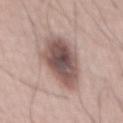Assessment: Recorded during total-body skin imaging; not selected for excision or biopsy. Clinical summary: A 15 mm crop from a total-body photograph taken for skin-cancer surveillance. A male subject aged 53 to 57.